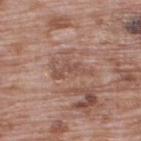  biopsy_status: not biopsied; imaged during a skin examination
  lesion_size:
    long_diameter_mm_approx: 4.5
  patient:
    sex: male
    age_approx: 70
  image:
    source: total-body photography crop
    field_of_view_mm: 15
  site: upper back
  lighting: white-light